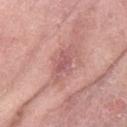Assessment:
Recorded during total-body skin imaging; not selected for excision or biopsy.
Acquisition and patient details:
The lesion is on the left forearm. Captured under white-light illumination. Approximately 5 mm at its widest. A female subject aged 68 to 72. A 15 mm close-up extracted from a 3D total-body photography capture.